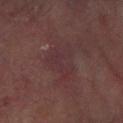Findings:
– notes: catalogued during a skin exam; not biopsied
– patient: female, aged approximately 80
– body site: the left arm
– TBP lesion metrics: a footprint of about 11 mm²; about 4 CIELAB-L* units darker than the surrounding skin
– size: about 4.5 mm
– acquisition: ~15 mm tile from a whole-body skin photo